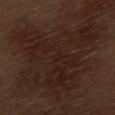notes = total-body-photography surveillance lesion; no biopsy
acquisition = total-body-photography crop, ~15 mm field of view
body site = the left thigh
patient = male, aged around 70
size = ≈12.5 mm
illumination = white-light illumination